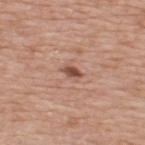The lesion was tiled from a total-body skin photograph and was not biopsied. Automated image analysis of the tile measured an eccentricity of roughly 0.85 and a symmetry-axis asymmetry near 0.35. The software also gave a lesion color around L≈50 a*≈23 b*≈28 in CIELAB, about 13 CIELAB-L* units darker than the surrounding skin, and a normalized lesion–skin contrast near 8.5. The software also gave a border-irregularity index near 3/10, a within-lesion color-variation index near 2/10, and a peripheral color-asymmetry measure near 0.5. The analysis additionally found an automated nevus-likeness rating near 75 out of 100 and a lesion-detection confidence of about 100/100. The lesion is on the upper back. About 2.5 mm across. A male subject aged 73–77. Captured under white-light illumination. A region of skin cropped from a whole-body photographic capture, roughly 15 mm wide.